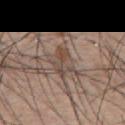A male subject aged approximately 55.
Measured at roughly 5 mm in maximum diameter.
Located on the back.
Imaged with white-light lighting.
A roughly 15 mm field-of-view crop from a total-body skin photograph.
The lesion-visualizer software estimated a shape eccentricity near 0.85. The software also gave a nevus-likeness score of about 0/100 and a detector confidence of about 50 out of 100 that the crop contains a lesion.